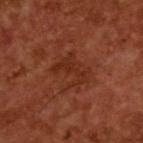Q: Is there a histopathology result?
A: no biopsy performed (imaged during a skin exam)
Q: What is the imaging modality?
A: total-body-photography crop, ~15 mm field of view
Q: Patient demographics?
A: male, aged around 65